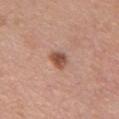follow-up — total-body-photography surveillance lesion; no biopsy
acquisition — ~15 mm tile from a whole-body skin photo
subject — male, aged 58–62
lesion size — ~2.5 mm (longest diameter)
lighting — white-light
anatomic site — the chest